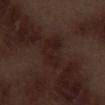No biopsy was performed on this lesion — it was imaged during a full skin examination and was not determined to be concerning.
About 4.5 mm across.
The subject is a male aged 68 to 72.
The lesion is on the leg.
A 15 mm close-up tile from a total-body photography series done for melanoma screening.
This is a white-light tile.
Automated tile analysis of the lesion measured a footprint of about 8.5 mm². And it measured roughly 5 lightness units darker than nearby skin. And it measured a nevus-likeness score of about 0/100 and a lesion-detection confidence of about 85/100.